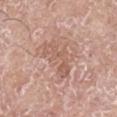<record>
  <biopsy_status>not biopsied; imaged during a skin examination</biopsy_status>
  <patient>
    <sex>male</sex>
    <age_approx>75</age_approx>
  </patient>
  <lighting>white-light</lighting>
  <site>right upper arm</site>
  <image>
    <source>total-body photography crop</source>
    <field_of_view_mm>15</field_of_view_mm>
  </image>
  <automated_metrics>
    <area_mm2_approx>10.0</area_mm2_approx>
    <shape_asymmetry>0.6</shape_asymmetry>
    <cielab_L>59</cielab_L>
    <cielab_a>20</cielab_a>
    <cielab_b>27</cielab_b>
    <vs_skin_darker_L>7.0</vs_skin_darker_L>
    <vs_skin_contrast_norm>5.0</vs_skin_contrast_norm>
  </automated_metrics>
</record>